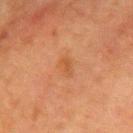Q: How large is the lesion?
A: ~3 mm (longest diameter)
Q: What is the imaging modality?
A: ~15 mm tile from a whole-body skin photo
Q: Lesion location?
A: the mid back
Q: Who is the patient?
A: male, aged 78–82
Q: How was the tile lit?
A: cross-polarized illumination